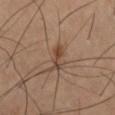Recorded during total-body skin imaging; not selected for excision or biopsy.
A male subject in their 60s.
The lesion-visualizer software estimated an area of roughly 4 mm², an eccentricity of roughly 0.95, and a symmetry-axis asymmetry near 0.35.
A region of skin cropped from a whole-body photographic capture, roughly 15 mm wide.
Imaged with cross-polarized lighting.
The lesion is on the right thigh.
Approximately 3.5 mm at its widest.